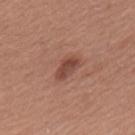Clinical impression:
Recorded during total-body skin imaging; not selected for excision or biopsy.
Clinical summary:
Captured under white-light illumination. A close-up tile cropped from a whole-body skin photograph, about 15 mm across. Measured at roughly 3.5 mm in maximum diameter. From the upper back. A male patient in their mid-60s.